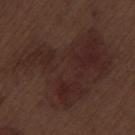follow-up — imaged on a skin check; not biopsied
anatomic site — the left thigh
patient — male, aged approximately 70
TBP lesion metrics — a border-irregularity rating of about 7/10, a within-lesion color-variation index near 4/10, and a peripheral color-asymmetry measure near 1; a nevus-likeness score of about 20/100 and a lesion-detection confidence of about 100/100
size — ≈13 mm
image source — total-body-photography crop, ~15 mm field of view